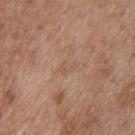Imaged with white-light lighting.
A male patient, roughly 50 years of age.
Located on the upper back.
A 15 mm crop from a total-body photograph taken for skin-cancer surveillance.